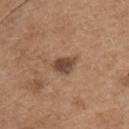<record>
<biopsy_status>not biopsied; imaged during a skin examination</biopsy_status>
<patient>
  <sex>male</sex>
  <age_approx>65</age_approx>
</patient>
<lesion_size>
  <long_diameter_mm_approx>3.0</long_diameter_mm_approx>
</lesion_size>
<automated_metrics>
  <nevus_likeness_0_100>80</nevus_likeness_0_100>
</automated_metrics>
<image>
  <source>total-body photography crop</source>
  <field_of_view_mm>15</field_of_view_mm>
</image>
<site>chest</site>
</record>